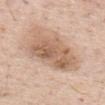<record>
  <image>
    <source>total-body photography crop</source>
    <field_of_view_mm>15</field_of_view_mm>
  </image>
  <patient>
    <sex>male</sex>
    <age_approx>55</age_approx>
  </patient>
  <site>back</site>
</record>